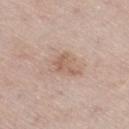Impression:
No biopsy was performed on this lesion — it was imaged during a full skin examination and was not determined to be concerning.
Background:
A 15 mm close-up tile from a total-body photography series done for melanoma screening. From the leg. A male subject, aged around 60. About 3 mm across. Imaged with white-light lighting.